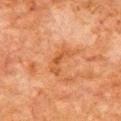Findings:
- workup — imaged on a skin check; not biopsied
- patient — male, approximately 80 years of age
- acquisition — ~15 mm tile from a whole-body skin photo
- lighting — cross-polarized illumination
- site — the chest